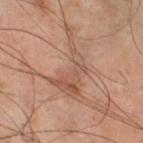Background: The lesion is on the left lower leg. Approximately 7.5 mm at its widest. Imaged with cross-polarized lighting. A male patient, in their 50s. A 15 mm close-up tile from a total-body photography series done for melanoma screening.